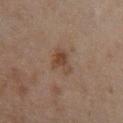Image and clinical context: Automated tile analysis of the lesion measured an area of roughly 5.5 mm², a shape eccentricity near 0.7, and a symmetry-axis asymmetry near 0.4. And it measured an average lesion color of about L≈37 a*≈15 b*≈25 (CIELAB) and roughly 7 lightness units darker than nearby skin. The software also gave a border-irregularity rating of about 4/10, internal color variation of about 2.5 on a 0–10 scale, and radial color variation of about 0.5. The software also gave a nevus-likeness score of about 60/100 and a detector confidence of about 100 out of 100 that the crop contains a lesion. Cropped from a total-body skin-imaging series; the visible field is about 15 mm. Approximately 3.5 mm at its widest. Imaged with cross-polarized lighting. Located on the right lower leg. A female subject, aged around 60.